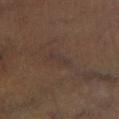This lesion was catalogued during total-body skin photography and was not selected for biopsy.
A 15 mm close-up tile from a total-body photography series done for melanoma screening.
The subject is a male about 50 years old.
The lesion is located on the right lower leg.
Measured at roughly 3 mm in maximum diameter.
The total-body-photography lesion software estimated a footprint of about 2.5 mm² and a shape-asymmetry score of about 0.4 (0 = symmetric). The analysis additionally found a mean CIELAB color near L≈33 a*≈13 b*≈20, about 3 CIELAB-L* units darker than the surrounding skin, and a lesion-to-skin contrast of about 4.5 (normalized; higher = more distinct). And it measured a classifier nevus-likeness of about 0/100.